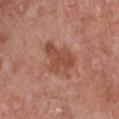body site: the front of the torso | patient: female, in their 60s | image source: ~15 mm crop, total-body skin-cancer survey | lesion diameter: ~4.5 mm (longest diameter) | image-analysis metrics: a lesion color around L≈49 a*≈25 b*≈30 in CIELAB, about 9 CIELAB-L* units darker than the surrounding skin, and a lesion-to-skin contrast of about 7 (normalized; higher = more distinct); border irregularity of about 4 on a 0–10 scale and peripheral color asymmetry of about 1; an automated nevus-likeness rating near 10 out of 100 and a detector confidence of about 100 out of 100 that the crop contains a lesion | tile lighting: white-light illumination.